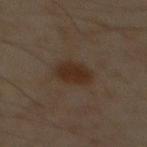<tbp_lesion>
  <biopsy_status>not biopsied; imaged during a skin examination</biopsy_status>
  <lighting>cross-polarized</lighting>
  <automated_metrics>
    <area_mm2_approx>7.0</area_mm2_approx>
    <eccentricity>0.65</eccentricity>
    <shape_asymmetry>0.15</shape_asymmetry>
    <cielab_L>25</cielab_L>
    <cielab_a>13</cielab_a>
    <cielab_b>23</cielab_b>
    <vs_skin_darker_L>7.0</vs_skin_darker_L>
    <vs_skin_contrast_norm>9.0</vs_skin_contrast_norm>
    <border_irregularity_0_10>1.5</border_irregularity_0_10>
    <color_variation_0_10>2.0</color_variation_0_10>
    <peripheral_color_asymmetry>0.5</peripheral_color_asymmetry>
    <nevus_likeness_0_100>85</nevus_likeness_0_100>
    <lesion_detection_confidence_0_100>100</lesion_detection_confidence_0_100>
  </automated_metrics>
  <image>
    <source>total-body photography crop</source>
    <field_of_view_mm>15</field_of_view_mm>
  </image>
  <site>chest</site>
  <patient>
    <sex>male</sex>
    <age_approx>60</age_approx>
  </patient>
</tbp_lesion>